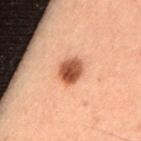Notes:
- workup: total-body-photography surveillance lesion; no biopsy